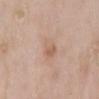The lesion was photographed on a routine skin check and not biopsied; there is no pathology result.